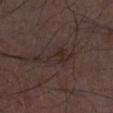Part of a total-body skin-imaging series; this lesion was reviewed on a skin check and was not flagged for biopsy. Cropped from a total-body skin-imaging series; the visible field is about 15 mm. Imaged with white-light lighting. The lesion-visualizer software estimated internal color variation of about 2 on a 0–10 scale and peripheral color asymmetry of about 0.5. And it measured a nevus-likeness score of about 0/100 and a detector confidence of about 60 out of 100 that the crop contains a lesion. Measured at roughly 5.5 mm in maximum diameter. A male patient aged around 50. The lesion is on the leg.